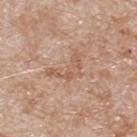notes: no biopsy performed (imaged during a skin exam); subject: male, aged 78–82; image source: total-body-photography crop, ~15 mm field of view; site: the back.